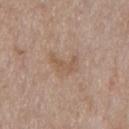notes = imaged on a skin check; not biopsied
acquisition = total-body-photography crop, ~15 mm field of view
tile lighting = white-light
subject = male, aged 53 to 57
site = the chest
automated lesion analysis = roughly 7 lightness units darker than nearby skin and a normalized lesion–skin contrast near 5.5; a classifier nevus-likeness of about 0/100 and a detector confidence of about 100 out of 100 that the crop contains a lesion